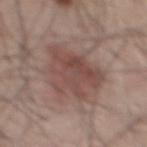Impression:
The lesion was photographed on a routine skin check and not biopsied; there is no pathology result.
Acquisition and patient details:
Longest diameter approximately 6.5 mm. A roughly 15 mm field-of-view crop from a total-body skin photograph. The lesion is located on the mid back. The tile uses white-light illumination. A male patient aged 48–52. The lesion-visualizer software estimated an area of roughly 20 mm², an eccentricity of roughly 0.7, and two-axis asymmetry of about 0.4. And it measured an average lesion color of about L≈47 a*≈19 b*≈22 (CIELAB) and roughly 9 lightness units darker than nearby skin.